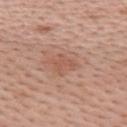Captured during whole-body skin photography for melanoma surveillance; the lesion was not biopsied.
A close-up tile cropped from a whole-body skin photograph, about 15 mm across.
The lesion is located on the upper back.
A female patient aged approximately 35.